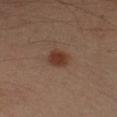– patient: male, about 50 years old
– anatomic site: the arm
– image source: ~15 mm crop, total-body skin-cancer survey